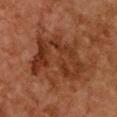Notes:
* automated metrics: a mean CIELAB color near L≈31 a*≈22 b*≈29, about 8 CIELAB-L* units darker than the surrounding skin, and a normalized lesion–skin contrast near 7.5; a classifier nevus-likeness of about 5/100 and a lesion-detection confidence of about 100/100
* acquisition: ~15 mm tile from a whole-body skin photo
* diameter: ≈8 mm
* subject: female, approximately 60 years of age
* anatomic site: the chest